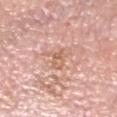Impression:
No biopsy was performed on this lesion — it was imaged during a full skin examination and was not determined to be concerning.
Background:
This is a white-light tile. Cropped from a total-body skin-imaging series; the visible field is about 15 mm. On the head or neck. A male subject in their 60s. Longest diameter approximately 3.5 mm.